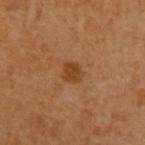Q: Was this lesion biopsied?
A: no biopsy performed (imaged during a skin exam)
Q: What lighting was used for the tile?
A: cross-polarized illumination
Q: What kind of image is this?
A: ~15 mm crop, total-body skin-cancer survey
Q: Lesion size?
A: about 2 mm
Q: Lesion location?
A: the left upper arm
Q: Patient demographics?
A: male, aged 58 to 62